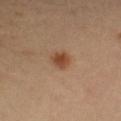Q: Was this lesion biopsied?
A: imaged on a skin check; not biopsied
Q: How was this image acquired?
A: ~15 mm crop, total-body skin-cancer survey
Q: Where on the body is the lesion?
A: the left forearm
Q: What are the patient's age and sex?
A: female, aged approximately 40